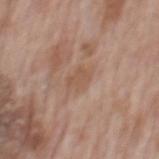Imaged during a routine full-body skin examination; the lesion was not biopsied and no histopathology is available. The lesion is located on the mid back. Approximately 3.5 mm at its widest. Automated image analysis of the tile measured a border-irregularity index near 3.5/10, a within-lesion color-variation index near 1.5/10, and radial color variation of about 0.5. The analysis additionally found an automated nevus-likeness rating near 0 out of 100. A 15 mm crop from a total-body photograph taken for skin-cancer surveillance. The subject is a male aged 68 to 72.